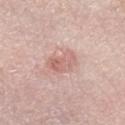notes=catalogued during a skin exam; not biopsied | size=≈4 mm | tile lighting=white-light | body site=the right lower leg | image source=total-body-photography crop, ~15 mm field of view | subject=female, approximately 65 years of age.